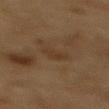automated lesion analysis: a lesion color around L≈30 a*≈13 b*≈26 in CIELAB, roughly 4 lightness units darker than nearby skin, and a normalized lesion–skin contrast near 5; a lesion-detection confidence of about 100/100 | location: the mid back | subject: male, in their mid- to late 80s or older | tile lighting: cross-polarized illumination | acquisition: ~15 mm crop, total-body skin-cancer survey | size: about 2.5 mm.